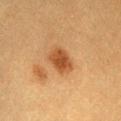Context: On the lower back. A 15 mm close-up tile from a total-body photography series done for melanoma screening. The lesion's longest dimension is about 3.5 mm. A female patient in their 40s. The lesion-visualizer software estimated a within-lesion color-variation index near 2.5/10 and a peripheral color-asymmetry measure near 1.